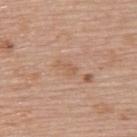The lesion was photographed on a routine skin check and not biopsied; there is no pathology result. The lesion is located on the upper back. Longest diameter approximately 2.5 mm. A roughly 15 mm field-of-view crop from a total-body skin photograph. Captured under white-light illumination. A female subject approximately 60 years of age.